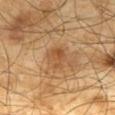Q: Was this lesion biopsied?
A: imaged on a skin check; not biopsied
Q: Lesion size?
A: ~3 mm (longest diameter)
Q: Lesion location?
A: the mid back
Q: What kind of image is this?
A: 15 mm crop, total-body photography
Q: Automated lesion metrics?
A: a mean CIELAB color near L≈49 a*≈20 b*≈36, a lesion–skin lightness drop of about 8, and a lesion-to-skin contrast of about 6 (normalized; higher = more distinct); a border-irregularity rating of about 3/10, internal color variation of about 4 on a 0–10 scale, and peripheral color asymmetry of about 1.5
Q: What are the patient's age and sex?
A: male, about 65 years old
Q: What lighting was used for the tile?
A: cross-polarized illumination